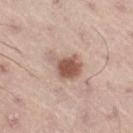Recorded during total-body skin imaging; not selected for excision or biopsy.
Captured under white-light illumination.
On the right thigh.
A male subject aged 53–57.
A region of skin cropped from a whole-body photographic capture, roughly 15 mm wide.
Approximately 4 mm at its widest.